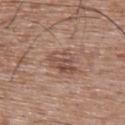workup: total-body-photography surveillance lesion; no biopsy
size: about 4 mm
illumination: white-light illumination
acquisition: ~15 mm tile from a whole-body skin photo
subject: male, aged 48 to 52
site: the upper back